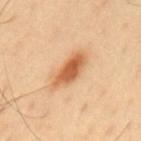Image and clinical context:
A region of skin cropped from a whole-body photographic capture, roughly 15 mm wide. Imaged with cross-polarized lighting. About 5.5 mm across. A male subject, about 40 years old. The lesion is located on the upper back.